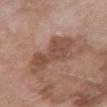No biopsy was performed on this lesion — it was imaged during a full skin examination and was not determined to be concerning. A lesion tile, about 15 mm wide, cut from a 3D total-body photograph. Longest diameter approximately 7 mm. A female patient roughly 70 years of age. From the front of the torso. The tile uses white-light illumination.